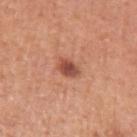Part of a total-body skin-imaging series; this lesion was reviewed on a skin check and was not flagged for biopsy. Automated image analysis of the tile measured a mean CIELAB color near L≈50 a*≈27 b*≈31 and a lesion-to-skin contrast of about 9 (normalized; higher = more distinct). A 15 mm crop from a total-body photograph taken for skin-cancer surveillance. Located on the arm. Longest diameter approximately 3 mm. The subject is a male aged 53 to 57.